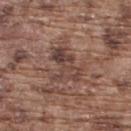Q: Was this lesion biopsied?
A: total-body-photography surveillance lesion; no biopsy
Q: How large is the lesion?
A: about 5 mm
Q: Who is the patient?
A: male, approximately 75 years of age
Q: How was the tile lit?
A: white-light illumination
Q: Lesion location?
A: the back
Q: What kind of image is this?
A: ~15 mm tile from a whole-body skin photo